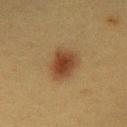This lesion was catalogued during total-body skin photography and was not selected for biopsy.
Captured under cross-polarized illumination.
A female patient, aged 38–42.
A 15 mm crop from a total-body photograph taken for skin-cancer surveillance.
Approximately 4.5 mm at its widest.
Located on the chest.